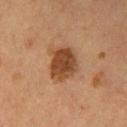Acquisition and patient details:
The lesion is on the chest. Imaged with cross-polarized lighting. A 15 mm close-up extracted from a 3D total-body photography capture. A male patient, in their mid- to late 50s. Measured at roughly 4.5 mm in maximum diameter.